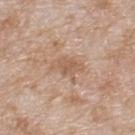follow-up — catalogued during a skin exam; not biopsied
tile lighting — white-light
site — the upper back
automated metrics — a shape eccentricity near 0.8 and a shape-asymmetry score of about 0.4 (0 = symmetric); a lesion color around L≈58 a*≈18 b*≈31 in CIELAB, about 8 CIELAB-L* units darker than the surrounding skin, and a normalized lesion–skin contrast near 6; lesion-presence confidence of about 100/100
acquisition — 15 mm crop, total-body photography
patient — male, aged around 80